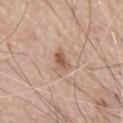follow-up: no biopsy performed (imaged during a skin exam); anatomic site: the chest; image: ~15 mm crop, total-body skin-cancer survey; patient: male, aged approximately 80; tile lighting: white-light illumination; lesion size: about 2.5 mm.